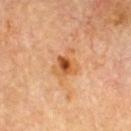Q: Was a biopsy performed?
A: catalogued during a skin exam; not biopsied
Q: Lesion location?
A: the chest
Q: Illumination type?
A: cross-polarized illumination
Q: What kind of image is this?
A: 15 mm crop, total-body photography
Q: Automated lesion metrics?
A: a nevus-likeness score of about 80/100 and lesion-presence confidence of about 100/100
Q: How large is the lesion?
A: about 4 mm
Q: What are the patient's age and sex?
A: male, about 60 years old